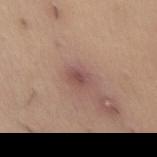Case summary:
* workup: no biopsy performed (imaged during a skin exam)
* patient: female, roughly 40 years of age
* location: the abdomen
* TBP lesion metrics: a lesion area of about 3.5 mm² and an outline eccentricity of about 0.7 (0 = round, 1 = elongated); a nevus-likeness score of about 25/100 and lesion-presence confidence of about 100/100
* illumination: white-light illumination
* acquisition: ~15 mm tile from a whole-body skin photo